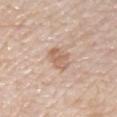Recorded during total-body skin imaging; not selected for excision or biopsy.
Longest diameter approximately 3 mm.
The lesion is on the chest.
A female patient in their mid-40s.
The tile uses white-light illumination.
A 15 mm close-up extracted from a 3D total-body photography capture.
Automated image analysis of the tile measured an area of roughly 5.5 mm². And it measured border irregularity of about 2.5 on a 0–10 scale and a within-lesion color-variation index near 3/10. The software also gave a classifier nevus-likeness of about 5/100 and lesion-presence confidence of about 100/100.